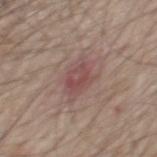  biopsy_status: not biopsied; imaged during a skin examination
  automated_metrics:
    border_irregularity_0_10: 2.5
    color_variation_0_10: 4.5
    peripheral_color_asymmetry: 1.5
  patient:
    sex: male
    age_approx: 70
  image:
    source: total-body photography crop
    field_of_view_mm: 15
  lighting: white-light
  site: mid back